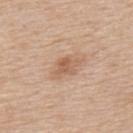workup: no biopsy performed (imaged during a skin exam) | body site: the upper back | image: ~15 mm crop, total-body skin-cancer survey | TBP lesion metrics: an area of roughly 4.5 mm² and an outline eccentricity of about 0.75 (0 = round, 1 = elongated); an automated nevus-likeness rating near 20 out of 100 | subject: male, aged approximately 60 | lighting: white-light illumination.